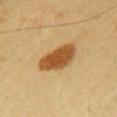Notes:
• biopsy status · imaged on a skin check; not biopsied
• acquisition · ~15 mm tile from a whole-body skin photo
• patient · female, aged around 30
• lesion size · ≈5.5 mm
• tile lighting · cross-polarized
• location · the chest